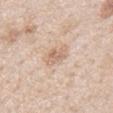workup = catalogued during a skin exam; not biopsied | TBP lesion metrics = a footprint of about 4.5 mm², an eccentricity of roughly 0.5, and a symmetry-axis asymmetry near 0.25 | patient = male, aged approximately 65 | lighting = white-light | body site = the chest | image source = total-body-photography crop, ~15 mm field of view.